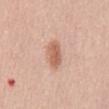{
  "biopsy_status": "not biopsied; imaged during a skin examination",
  "image": {
    "source": "total-body photography crop",
    "field_of_view_mm": 15
  },
  "lighting": "white-light",
  "patient": {
    "sex": "male",
    "age_approx": 55
  },
  "lesion_size": {
    "long_diameter_mm_approx": 4.0
  },
  "site": "abdomen",
  "automated_metrics": {
    "nevus_likeness_0_100": 95,
    "lesion_detection_confidence_0_100": 100
  }
}